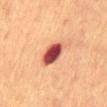Clinical impression: The lesion was photographed on a routine skin check and not biopsied; there is no pathology result. Image and clinical context: This is a cross-polarized tile. A male patient, aged 58–62. This image is a 15 mm lesion crop taken from a total-body photograph. Located on the front of the torso.